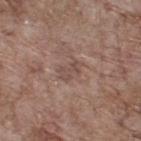lighting: white-light illumination | patient: male, roughly 70 years of age | location: the upper back | image: total-body-photography crop, ~15 mm field of view.